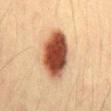No biopsy was performed on this lesion — it was imaged during a full skin examination and was not determined to be concerning. A male patient roughly 40 years of age. Approximately 6.5 mm at its widest. The lesion is located on the abdomen. A roughly 15 mm field-of-view crop from a total-body skin photograph. This is a cross-polarized tile. Automated image analysis of the tile measured a lesion color around L≈45 a*≈24 b*≈31 in CIELAB, roughly 23 lightness units darker than nearby skin, and a normalized border contrast of about 15.5. And it measured a border-irregularity index near 1.5/10 and radial color variation of about 2. And it measured lesion-presence confidence of about 100/100.